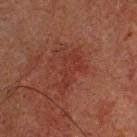The lesion was photographed on a routine skin check and not biopsied; there is no pathology result. A 15 mm close-up tile from a total-body photography series done for melanoma screening. Captured under cross-polarized illumination. The lesion is located on the head or neck. A male subject, about 60 years old. Automated image analysis of the tile measured an area of roughly 9 mm², an eccentricity of roughly 0.85, and a symmetry-axis asymmetry near 0.6. It also reported a mean CIELAB color near L≈26 a*≈22 b*≈22, roughly 4 lightness units darker than nearby skin, and a normalized lesion–skin contrast near 5. The software also gave border irregularity of about 9 on a 0–10 scale and a peripheral color-asymmetry measure near 0.5. It also reported lesion-presence confidence of about 100/100.